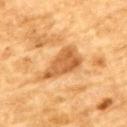Clinical impression: No biopsy was performed on this lesion — it was imaged during a full skin examination and was not determined to be concerning. Context: Captured under cross-polarized illumination. Approximately 6 mm at its widest. A region of skin cropped from a whole-body photographic capture, roughly 15 mm wide. On the upper back. A male patient aged approximately 85 or older.